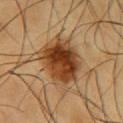Recorded during total-body skin imaging; not selected for excision or biopsy. A 15 mm crop from a total-body photograph taken for skin-cancer surveillance. The lesion-visualizer software estimated an area of roughly 24 mm² and two-axis asymmetry of about 0.2. And it measured an average lesion color of about L≈43 a*≈21 b*≈36 (CIELAB), roughly 15 lightness units darker than nearby skin, and a lesion-to-skin contrast of about 11.5 (normalized; higher = more distinct). The software also gave a color-variation rating of about 9.5/10 and a peripheral color-asymmetry measure near 3. It also reported lesion-presence confidence of about 100/100. On the chest. Longest diameter approximately 6.5 mm. Imaged with cross-polarized lighting. The patient is a male about 60 years old.